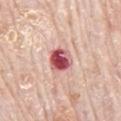Clinical impression:
This lesion was catalogued during total-body skin photography and was not selected for biopsy.
Background:
The tile uses white-light illumination. A male patient, aged 78–82. From the mid back. Approximately 3 mm at its widest. A 15 mm close-up extracted from a 3D total-body photography capture.